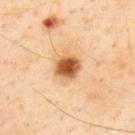Notes:
• biopsy status: catalogued during a skin exam; not biopsied
• subject: male, aged approximately 55
• location: the upper back
• lighting: cross-polarized illumination
• image source: total-body-photography crop, ~15 mm field of view
• lesion size: about 3 mm